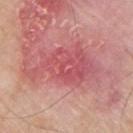notes: no biopsy performed (imaged during a skin exam); anatomic site: the chest; subject: male, about 55 years old; image source: ~15 mm crop, total-body skin-cancer survey.